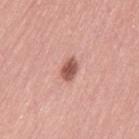biopsy status: total-body-photography surveillance lesion; no biopsy | lesion size: about 2.5 mm | body site: the left thigh | subject: female, aged approximately 65 | image: ~15 mm crop, total-body skin-cancer survey | illumination: white-light | automated metrics: a footprint of about 4 mm², a shape eccentricity near 0.75, and a symmetry-axis asymmetry near 0.15.